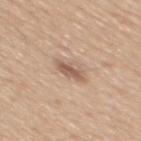– biopsy status: total-body-photography surveillance lesion; no biopsy
– lighting: white-light
– imaging modality: total-body-photography crop, ~15 mm field of view
– anatomic site: the mid back
– diameter: about 3.5 mm
– patient: male, approximately 50 years of age